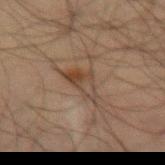workup = total-body-photography surveillance lesion; no biopsy
subject = male, about 70 years old
acquisition = ~15 mm tile from a whole-body skin photo
site = the abdomen
lesion size = ~4.5 mm (longest diameter)